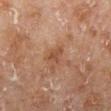* follow-up — catalogued during a skin exam; not biopsied
* illumination — cross-polarized
* diameter — about 2.5 mm
* body site — the right lower leg
* acquisition — ~15 mm tile from a whole-body skin photo
* TBP lesion metrics — an average lesion color of about L≈39 a*≈19 b*≈27 (CIELAB) and a lesion–skin lightness drop of about 7; a nevus-likeness score of about 0/100 and lesion-presence confidence of about 100/100
* subject — male, aged approximately 70